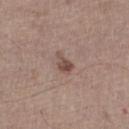Q: Was this lesion biopsied?
A: total-body-photography surveillance lesion; no biopsy
Q: What is the anatomic site?
A: the right lower leg
Q: Who is the patient?
A: female, in their mid-60s
Q: How was the tile lit?
A: white-light
Q: How was this image acquired?
A: total-body-photography crop, ~15 mm field of view